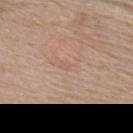Case summary:
– imaging modality — ~15 mm tile from a whole-body skin photo
– anatomic site — the upper back
– patient — female, aged around 65